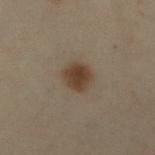Impression:
Captured during whole-body skin photography for melanoma surveillance; the lesion was not biopsied.
Background:
The lesion is located on the right lower leg. A roughly 15 mm field-of-view crop from a total-body skin photograph. Automated image analysis of the tile measured an average lesion color of about L≈34 a*≈12 b*≈23 (CIELAB), roughly 9 lightness units darker than nearby skin, and a lesion-to-skin contrast of about 9 (normalized; higher = more distinct). And it measured a border-irregularity index near 1.5/10, a within-lesion color-variation index near 2.5/10, and radial color variation of about 0.5. The analysis additionally found a classifier nevus-likeness of about 100/100 and a detector confidence of about 100 out of 100 that the crop contains a lesion. The patient is a female aged 48 to 52. The tile uses cross-polarized illumination.